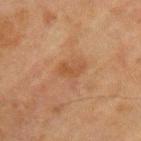This lesion was catalogued during total-body skin photography and was not selected for biopsy. An algorithmic analysis of the crop reported an eccentricity of roughly 0.85 and two-axis asymmetry of about 0.45. And it measured a lesion color around L≈41 a*≈19 b*≈32 in CIELAB, a lesion–skin lightness drop of about 6, and a lesion-to-skin contrast of about 6 (normalized; higher = more distinct). It also reported an automated nevus-likeness rating near 0 out of 100 and a lesion-detection confidence of about 100/100. Measured at roughly 2.5 mm in maximum diameter. This is a cross-polarized tile. A 15 mm close-up tile from a total-body photography series done for melanoma screening. A male subject, approximately 70 years of age. On the upper back.